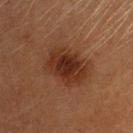biopsy status: imaged on a skin check; not biopsied | subject: female, aged around 40 | TBP lesion metrics: a footprint of about 19 mm² and a symmetry-axis asymmetry near 0.15; a mean CIELAB color near L≈26 a*≈19 b*≈25 and a lesion-to-skin contrast of about 8.5 (normalized; higher = more distinct); border irregularity of about 2.5 on a 0–10 scale, a color-variation rating of about 4.5/10, and radial color variation of about 1; an automated nevus-likeness rating near 90 out of 100 and lesion-presence confidence of about 100/100 | anatomic site: the head or neck | lesion size: ~5.5 mm (longest diameter) | illumination: cross-polarized illumination | image: ~15 mm crop, total-body skin-cancer survey.